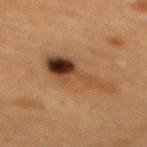Part of a total-body skin-imaging series; this lesion was reviewed on a skin check and was not flagged for biopsy.
From the mid back.
A female patient, about 60 years old.
Cropped from a whole-body photographic skin survey; the tile spans about 15 mm.